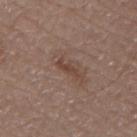Recorded during total-body skin imaging; not selected for excision or biopsy.
A male patient, aged 78–82.
A 15 mm crop from a total-body photograph taken for skin-cancer surveillance.
On the mid back.